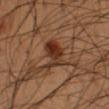{"biopsy_status": "not biopsied; imaged during a skin examination", "site": "left forearm", "image": {"source": "total-body photography crop", "field_of_view_mm": 15}, "automated_metrics": {"area_mm2_approx": 8.5, "shape_asymmetry": 0.35, "cielab_L": 26, "cielab_a": 17, "cielab_b": 24, "vs_skin_contrast_norm": 9.5, "border_irregularity_0_10": 4.0, "color_variation_0_10": 5.0, "lesion_detection_confidence_0_100": 100}, "patient": {"sex": "male", "age_approx": 55}, "lighting": "cross-polarized", "lesion_size": {"long_diameter_mm_approx": 3.5}}